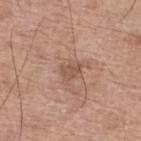This lesion was catalogued during total-body skin photography and was not selected for biopsy.
A region of skin cropped from a whole-body photographic capture, roughly 15 mm wide.
The tile uses white-light illumination.
From the left lower leg.
Longest diameter approximately 2.5 mm.
A male patient in their mid- to late 60s.